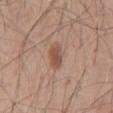* workup — catalogued during a skin exam; not biopsied
* lesion size — ≈3.5 mm
* subject — male, roughly 45 years of age
* image — 15 mm crop, total-body photography
* illumination — white-light
* body site — the lower back